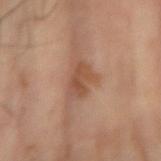Imaged during a routine full-body skin examination; the lesion was not biopsied and no histopathology is available.
The lesion is on the right forearm.
This image is a 15 mm lesion crop taken from a total-body photograph.
The tile uses cross-polarized illumination.
Measured at roughly 3.5 mm in maximum diameter.
A male subject about 70 years old.